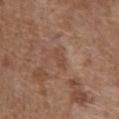{"biopsy_status": "not biopsied; imaged during a skin examination", "automated_metrics": {"area_mm2_approx": 2.5, "eccentricity": 0.9, "shape_asymmetry": 0.35, "cielab_L": 46, "cielab_a": 19, "cielab_b": 29, "vs_skin_darker_L": 6.0, "vs_skin_contrast_norm": 5.0, "color_variation_0_10": 0.0, "peripheral_color_asymmetry": 0.0, "nevus_likeness_0_100": 0, "lesion_detection_confidence_0_100": 100}, "image": {"source": "total-body photography crop", "field_of_view_mm": 15}, "site": "abdomen", "lighting": "white-light", "patient": {"sex": "male", "age_approx": 75}}